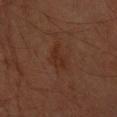| field | value |
|---|---|
| biopsy status | catalogued during a skin exam; not biopsied |
| body site | the right forearm |
| patient | male, aged approximately 60 |
| image source | 15 mm crop, total-body photography |